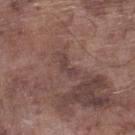Assessment:
Part of a total-body skin-imaging series; this lesion was reviewed on a skin check and was not flagged for biopsy.
Context:
Captured under white-light illumination. Cropped from a total-body skin-imaging series; the visible field is about 15 mm. A male patient, approximately 75 years of age. Approximately 3 mm at its widest. The lesion is located on the left lower leg.